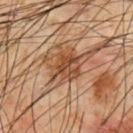automated_metrics:
  nevus_likeness_0_100: 0
lesion_size:
  long_diameter_mm_approx: 3.5
image:
  source: total-body photography crop
  field_of_view_mm: 15
patient:
  sex: male
  age_approx: 65
site: chest